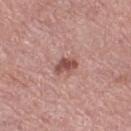Recorded during total-body skin imaging; not selected for excision or biopsy. Cropped from a total-body skin-imaging series; the visible field is about 15 mm. Located on the leg. The patient is a female approximately 55 years of age.